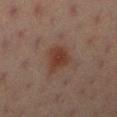Q: Was a biopsy performed?
A: catalogued during a skin exam; not biopsied
Q: Patient demographics?
A: female, aged 18–22
Q: What kind of image is this?
A: ~15 mm tile from a whole-body skin photo
Q: What is the anatomic site?
A: the right lower leg
Q: Lesion size?
A: about 3.5 mm